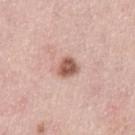This lesion was catalogued during total-body skin photography and was not selected for biopsy. From the arm. About 2.5 mm across. This image is a 15 mm lesion crop taken from a total-body photograph. The lesion-visualizer software estimated a lesion area of about 5 mm², an eccentricity of roughly 0.35, and a shape-asymmetry score of about 0.2 (0 = symmetric). The analysis additionally found a mean CIELAB color near L≈57 a*≈22 b*≈27, roughly 15 lightness units darker than nearby skin, and a lesion-to-skin contrast of about 10 (normalized; higher = more distinct). The software also gave a border-irregularity rating of about 2/10, internal color variation of about 4 on a 0–10 scale, and radial color variation of about 1.5. It also reported a nevus-likeness score of about 85/100. A male patient in their mid-40s. Imaged with white-light lighting.